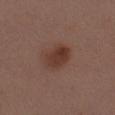Case summary:
• follow-up: total-body-photography surveillance lesion; no biopsy
• lighting: white-light
• body site: the right thigh
• automated lesion analysis: a mean CIELAB color near L≈36 a*≈20 b*≈24, roughly 9 lightness units darker than nearby skin, and a normalized border contrast of about 8; a border-irregularity rating of about 2/10, a color-variation rating of about 4/10, and peripheral color asymmetry of about 1.5; a classifier nevus-likeness of about 95/100 and lesion-presence confidence of about 100/100
• image: ~15 mm crop, total-body skin-cancer survey
• subject: female, aged 38–42
• size: ≈4 mm